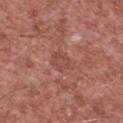No biopsy was performed on this lesion — it was imaged during a full skin examination and was not determined to be concerning.